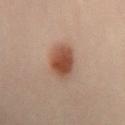{"biopsy_status": "not biopsied; imaged during a skin examination", "automated_metrics": {"border_irregularity_0_10": 2.0, "color_variation_0_10": 4.0, "nevus_likeness_0_100": 100, "lesion_detection_confidence_0_100": 100}, "site": "left forearm", "lesion_size": {"long_diameter_mm_approx": 5.0}, "patient": {"sex": "female", "age_approx": 30}, "image": {"source": "total-body photography crop", "field_of_view_mm": 15}}